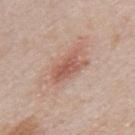lighting: white-light
automated_metrics:
  nevus_likeness_0_100: 60
  lesion_detection_confidence_0_100: 100
image:
  source: total-body photography crop
  field_of_view_mm: 15
patient:
  sex: male
  age_approx: 40
site: upper back